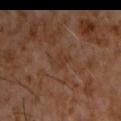{
  "biopsy_status": "not biopsied; imaged during a skin examination",
  "site": "chest",
  "image": {
    "source": "total-body photography crop",
    "field_of_view_mm": 15
  },
  "patient": {
    "sex": "male",
    "age_approx": 60
  }
}